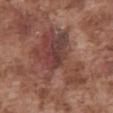A male subject, aged 73 to 77. Cropped from a whole-body photographic skin survey; the tile spans about 15 mm. The lesion is located on the front of the torso.